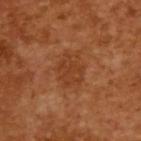| key | value |
|---|---|
| workup | catalogued during a skin exam; not biopsied |